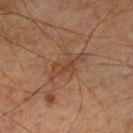Recorded during total-body skin imaging; not selected for excision or biopsy. This image is a 15 mm lesion crop taken from a total-body photograph. A male patient aged 68–72. The lesion is on the left lower leg.